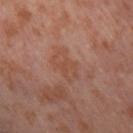follow-up=imaged on a skin check; not biopsied | subject=female, in their mid-50s | acquisition=total-body-photography crop, ~15 mm field of view | site=the right thigh | lesion diameter=~4 mm (longest diameter).